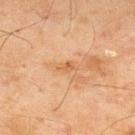Clinical impression: Imaged during a routine full-body skin examination; the lesion was not biopsied and no histopathology is available. Background: The recorded lesion diameter is about 2.5 mm. The lesion is located on the upper back. A roughly 15 mm field-of-view crop from a total-body skin photograph. Automated image analysis of the tile measured a symmetry-axis asymmetry near 0.3. And it measured border irregularity of about 3 on a 0–10 scale and radial color variation of about 0.5. This is a cross-polarized tile. The patient is a male in their mid- to late 40s.